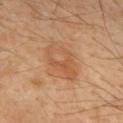{
  "biopsy_status": "not biopsied; imaged during a skin examination",
  "lesion_size": {
    "long_diameter_mm_approx": 5.0
  },
  "image": {
    "source": "total-body photography crop",
    "field_of_view_mm": 15
  },
  "site": "upper back",
  "patient": {
    "sex": "male",
    "age_approx": 50
  },
  "lighting": "cross-polarized"
}